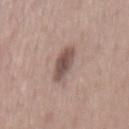follow-up: catalogued during a skin exam; not biopsied | lesion diameter: ≈4 mm | anatomic site: the back | imaging modality: 15 mm crop, total-body photography | patient: female, about 50 years old | tile lighting: white-light illumination.